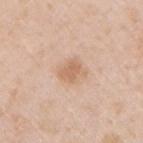Clinical impression: The lesion was photographed on a routine skin check and not biopsied; there is no pathology result.